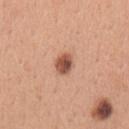{"biopsy_status": "not biopsied; imaged during a skin examination", "image": {"source": "total-body photography crop", "field_of_view_mm": 15}, "site": "left upper arm", "lesion_size": {"long_diameter_mm_approx": 2.5}, "patient": {"sex": "female", "age_approx": 30}, "lighting": "white-light", "automated_metrics": {"area_mm2_approx": 5.5, "shape_asymmetry": 0.15, "cielab_L": 54, "cielab_a": 24, "cielab_b": 30, "vs_skin_contrast_norm": 9.5}}